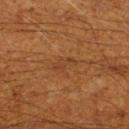<lesion>
<biopsy_status>not biopsied; imaged during a skin examination</biopsy_status>
<image>
  <source>total-body photography crop</source>
  <field_of_view_mm>15</field_of_view_mm>
</image>
<lesion_size>
  <long_diameter_mm_approx>3.0</long_diameter_mm_approx>
</lesion_size>
<site>leg</site>
<patient>
  <sex>male</sex>
  <age_approx>60</age_approx>
</patient>
</lesion>